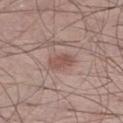Clinical impression: Part of a total-body skin-imaging series; this lesion was reviewed on a skin check and was not flagged for biopsy. Background: Approximately 3 mm at its widest. A male patient, in their mid-50s. From the right lower leg. Imaged with white-light lighting. A close-up tile cropped from a whole-body skin photograph, about 15 mm across.